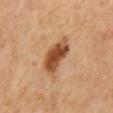The lesion was photographed on a routine skin check and not biopsied; there is no pathology result. The patient is a male about 60 years old. This is a cross-polarized tile. A 15 mm crop from a total-body photograph taken for skin-cancer surveillance. The recorded lesion diameter is about 4.5 mm. Located on the mid back. An algorithmic analysis of the crop reported a lesion color around L≈47 a*≈23 b*≈35 in CIELAB, a lesion–skin lightness drop of about 15, and a normalized lesion–skin contrast near 11. It also reported border irregularity of about 2.5 on a 0–10 scale, a within-lesion color-variation index near 5/10, and radial color variation of about 1.5. It also reported lesion-presence confidence of about 100/100.